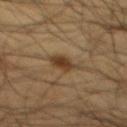biopsy status=total-body-photography surveillance lesion; no biopsy
image=15 mm crop, total-body photography
site=the front of the torso
automated metrics=internal color variation of about 2.5 on a 0–10 scale
lesion diameter=about 3 mm
illumination=cross-polarized
subject=male, approximately 60 years of age